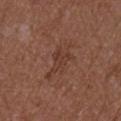The lesion was photographed on a routine skin check and not biopsied; there is no pathology result. The recorded lesion diameter is about 5.5 mm. The lesion is on the right upper arm. A male subject in their mid-50s. Imaged with white-light lighting. A close-up tile cropped from a whole-body skin photograph, about 15 mm across.